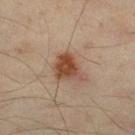Findings:
– workup — imaged on a skin check; not biopsied
– lesion size — ≈4 mm
– patient — male, aged approximately 45
– illumination — cross-polarized
– location — the right lower leg
– acquisition — 15 mm crop, total-body photography